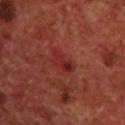| feature | finding |
|---|---|
| subject | male, aged 68 to 72 |
| diameter | ~3 mm (longest diameter) |
| acquisition | 15 mm crop, total-body photography |
| body site | the upper back |